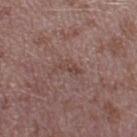image:
  source: total-body photography crop
  field_of_view_mm: 15
patient:
  sex: male
  age_approx: 40
lesion_size:
  long_diameter_mm_approx: 3.5
automated_metrics:
  cielab_L: 44
  cielab_a: 18
  cielab_b: 21
  vs_skin_darker_L: 6.0
  vs_skin_contrast_norm: 5.5
site: left thigh
lighting: white-light